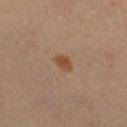biopsy status: catalogued during a skin exam; not biopsied
subject: female, aged 38–42
site: the right thigh
acquisition: 15 mm crop, total-body photography
size: ≈2.5 mm
automated lesion analysis: a lesion-to-skin contrast of about 8 (normalized; higher = more distinct); a within-lesion color-variation index near 1.5/10 and a peripheral color-asymmetry measure near 0.5